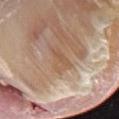The lesion was tiled from a total-body skin photograph and was not biopsied. The patient is a male roughly 80 years of age. From the right lower leg. A 15 mm close-up extracted from a 3D total-body photography capture.